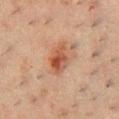No biopsy was performed on this lesion — it was imaged during a full skin examination and was not determined to be concerning. This image is a 15 mm lesion crop taken from a total-body photograph. Measured at roughly 3.5 mm in maximum diameter. This is a cross-polarized tile. A male subject aged 48–52. The lesion is on the chest.